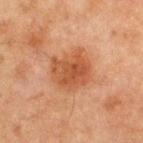The lesion was tiled from a total-body skin photograph and was not biopsied.
The total-body-photography lesion software estimated a footprint of about 16 mm², an outline eccentricity of about 0.4 (0 = round, 1 = elongated), and a shape-asymmetry score of about 0.2 (0 = symmetric). It also reported a border-irregularity index near 2.5/10 and internal color variation of about 4.5 on a 0–10 scale. It also reported a nevus-likeness score of about 35/100.
On the chest.
Imaged with cross-polarized lighting.
Measured at roughly 5 mm in maximum diameter.
A male subject roughly 65 years of age.
A close-up tile cropped from a whole-body skin photograph, about 15 mm across.